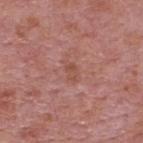Q: How was the tile lit?
A: white-light
Q: Lesion location?
A: the upper back
Q: What is the imaging modality?
A: total-body-photography crop, ~15 mm field of view
Q: What is the lesion's diameter?
A: about 3 mm
Q: What did automated image analysis measure?
A: a mean CIELAB color near L≈51 a*≈24 b*≈27 and about 6 CIELAB-L* units darker than the surrounding skin; a border-irregularity index near 4.5/10 and a peripheral color-asymmetry measure near 1; a nevus-likeness score of about 0/100 and lesion-presence confidence of about 100/100
Q: What are the patient's age and sex?
A: male, approximately 75 years of age